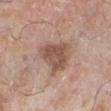workup = catalogued during a skin exam; not biopsied
size = ~5 mm (longest diameter)
image = ~15 mm crop, total-body skin-cancer survey
subject = male, aged 78–82
tile lighting = white-light illumination
anatomic site = the left lower leg